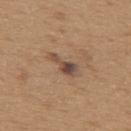Clinical summary: Approximately 4 mm at its widest. A 15 mm crop from a total-body photograph taken for skin-cancer surveillance. A male subject in their mid-60s. Imaged with white-light lighting. From the upper back. The total-body-photography lesion software estimated an area of roughly 4.5 mm² and an outline eccentricity of about 0.9 (0 = round, 1 = elongated). The analysis additionally found a mean CIELAB color near L≈47 a*≈17 b*≈26 and a lesion-to-skin contrast of about 9.5 (normalized; higher = more distinct). The analysis additionally found border irregularity of about 5.5 on a 0–10 scale, internal color variation of about 5 on a 0–10 scale, and peripheral color asymmetry of about 1. The software also gave a nevus-likeness score of about 15/100 and lesion-presence confidence of about 100/100.